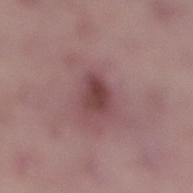follow-up: imaged on a skin check; not biopsied
lesion size: ~3.5 mm (longest diameter)
subject: male, aged 48 to 52
image source: ~15 mm tile from a whole-body skin photo
anatomic site: the right lower leg
TBP lesion metrics: two-axis asymmetry of about 0.3
tile lighting: white-light illumination